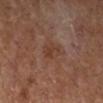Impression: This lesion was catalogued during total-body skin photography and was not selected for biopsy. Image and clinical context: Cropped from a total-body skin-imaging series; the visible field is about 15 mm. The tile uses cross-polarized illumination. Located on the right lower leg. A female subject. Measured at roughly 3 mm in maximum diameter.